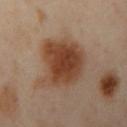Imaged during a routine full-body skin examination; the lesion was not biopsied and no histopathology is available.
A roughly 15 mm field-of-view crop from a total-body skin photograph.
A female patient, aged approximately 40.
Measured at roughly 6 mm in maximum diameter.
The total-body-photography lesion software estimated a lesion area of about 25 mm² and a shape eccentricity near 0.45. The analysis additionally found a lesion color around L≈43 a*≈20 b*≈31 in CIELAB and a lesion–skin lightness drop of about 13. The software also gave a border-irregularity rating of about 3/10 and a color-variation rating of about 4.5/10. And it measured a nevus-likeness score of about 100/100 and lesion-presence confidence of about 100/100.
Imaged with cross-polarized lighting.
The lesion is on the left arm.